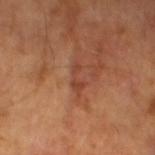<tbp_lesion>
<biopsy_status>not biopsied; imaged during a skin examination</biopsy_status>
<automated_metrics>
  <eccentricity>0.95</eccentricity>
  <shape_asymmetry>0.4</shape_asymmetry>
  <cielab_L>42</cielab_L>
  <cielab_a>25</cielab_a>
  <cielab_b>30</cielab_b>
  <vs_skin_darker_L>7.0</vs_skin_darker_L>
  <vs_skin_contrast_norm>6.0</vs_skin_contrast_norm>
  <lesion_detection_confidence_0_100>95</lesion_detection_confidence_0_100>
</automated_metrics>
<patient>
  <sex>male</sex>
  <age_approx>65</age_approx>
</patient>
<image>
  <source>total-body photography crop</source>
  <field_of_view_mm>15</field_of_view_mm>
</image>
<lighting>cross-polarized</lighting>
<lesion_size>
  <long_diameter_mm_approx>4.0</long_diameter_mm_approx>
</lesion_size>
</tbp_lesion>